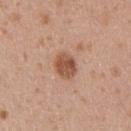Recorded during total-body skin imaging; not selected for excision or biopsy. Captured under white-light illumination. About 3.5 mm across. The lesion is located on the right upper arm. A lesion tile, about 15 mm wide, cut from a 3D total-body photograph. A male patient, about 35 years old.